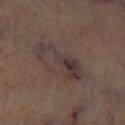Notes:
• workup: no biopsy performed (imaged during a skin exam)
• body site: the left lower leg
• image: ~15 mm tile from a whole-body skin photo
• illumination: cross-polarized illumination
• diameter: ≈6.5 mm
• image-analysis metrics: a lesion area of about 12 mm², a shape eccentricity near 0.9, and a shape-asymmetry score of about 0.55 (0 = symmetric); a lesion-to-skin contrast of about 7.5 (normalized; higher = more distinct); a border-irregularity rating of about 8/10, a within-lesion color-variation index near 6/10, and radial color variation of about 2; an automated nevus-likeness rating near 0 out of 100 and a lesion-detection confidence of about 95/100